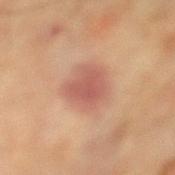The lesion was photographed on a routine skin check and not biopsied; there is no pathology result.
A close-up tile cropped from a whole-body skin photograph, about 15 mm across.
The recorded lesion diameter is about 4 mm.
This is a cross-polarized tile.
Automated image analysis of the tile measured a lesion color around L≈49 a*≈22 b*≈25 in CIELAB, roughly 9 lightness units darker than nearby skin, and a normalized border contrast of about 7. And it measured a border-irregularity index near 2.5/10 and radial color variation of about 0.5.
The subject is a female in their mid-50s.
On the left lower leg.